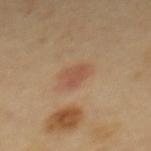  biopsy_status: not biopsied; imaged during a skin examination
  image:
    source: total-body photography crop
    field_of_view_mm: 15
  site: upper back
  lesion_size:
    long_diameter_mm_approx: 2.5
  automated_metrics:
    area_mm2_approx: 3.5
    cielab_L: 51
    cielab_a: 22
    cielab_b: 32
    vs_skin_darker_L: 8.0
    vs_skin_contrast_norm: 5.5
    nevus_likeness_0_100: 85
    lesion_detection_confidence_0_100: 100
  patient:
    sex: female
    age_approx: 60
  lighting: cross-polarized